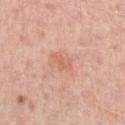Assessment:
Imaged during a routine full-body skin examination; the lesion was not biopsied and no histopathology is available.
Context:
An algorithmic analysis of the crop reported a footprint of about 3 mm². And it measured a nevus-likeness score of about 10/100 and lesion-presence confidence of about 100/100. Captured under white-light illumination. On the left forearm. A 15 mm close-up extracted from a 3D total-body photography capture. The patient is a female roughly 45 years of age.